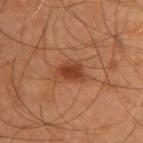Clinical impression: The lesion was tiled from a total-body skin photograph and was not biopsied. Acquisition and patient details: The lesion is located on the upper back. Captured under cross-polarized illumination. An algorithmic analysis of the crop reported a within-lesion color-variation index near 3/10. A 15 mm crop from a total-body photograph taken for skin-cancer surveillance. About 3.5 mm across. A male subject, aged approximately 55.